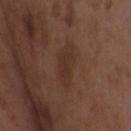Q: Was this lesion biopsied?
A: no biopsy performed (imaged during a skin exam)
Q: What are the patient's age and sex?
A: female, approximately 35 years of age
Q: Automated lesion metrics?
A: an eccentricity of roughly 0.85 and a shape-asymmetry score of about 0.25 (0 = symmetric); a mean CIELAB color near L≈32 a*≈18 b*≈24 and a lesion–skin lightness drop of about 5
Q: What is the anatomic site?
A: the upper back
Q: How was this image acquired?
A: total-body-photography crop, ~15 mm field of view
Q: Illumination type?
A: white-light
Q: How large is the lesion?
A: ~4.5 mm (longest diameter)